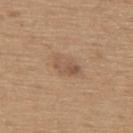<record>
<biopsy_status>not biopsied; imaged during a skin examination</biopsy_status>
<site>upper back</site>
<image>
  <source>total-body photography crop</source>
  <field_of_view_mm>15</field_of_view_mm>
</image>
<patient>
  <sex>male</sex>
  <age_approx>65</age_approx>
</patient>
</record>